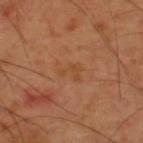Q: Was a biopsy performed?
A: total-body-photography surveillance lesion; no biopsy
Q: What kind of image is this?
A: ~15 mm tile from a whole-body skin photo
Q: What did automated image analysis measure?
A: border irregularity of about 5.5 on a 0–10 scale and a within-lesion color-variation index near 0.5/10; a classifier nevus-likeness of about 0/100 and a detector confidence of about 100 out of 100 that the crop contains a lesion
Q: What are the patient's age and sex?
A: male, about 60 years old
Q: How large is the lesion?
A: ~2.5 mm (longest diameter)
Q: How was the tile lit?
A: cross-polarized
Q: Where on the body is the lesion?
A: the upper back